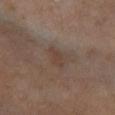Recorded during total-body skin imaging; not selected for excision or biopsy. From the right leg. A 15 mm crop from a total-body photograph taken for skin-cancer surveillance. A female subject roughly 65 years of age.